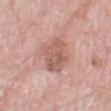biopsy status: catalogued during a skin exam; not biopsied
body site: the right thigh
illumination: white-light
imaging modality: total-body-photography crop, ~15 mm field of view
lesion size: ~5 mm (longest diameter)
automated metrics: an area of roughly 13 mm², an outline eccentricity of about 0.65 (0 = round, 1 = elongated), and two-axis asymmetry of about 0.2; a border-irregularity rating of about 2.5/10, internal color variation of about 4.5 on a 0–10 scale, and peripheral color asymmetry of about 1.5
subject: male, about 80 years old